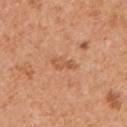The lesion was tiled from a total-body skin photograph and was not biopsied. This is a white-light tile. A 15 mm close-up extracted from a 3D total-body photography capture. An algorithmic analysis of the crop reported a footprint of about 3.5 mm² and two-axis asymmetry of about 0.3. The analysis additionally found a lesion–skin lightness drop of about 8. The lesion is located on the chest. The lesion's longest dimension is about 3.5 mm. A male subject, approximately 55 years of age.